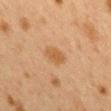{
  "biopsy_status": "not biopsied; imaged during a skin examination",
  "site": "back",
  "patient": {
    "sex": "female",
    "age_approx": 40
  },
  "image": {
    "source": "total-body photography crop",
    "field_of_view_mm": 15
  },
  "lesion_size": {
    "long_diameter_mm_approx": 3.5
  },
  "lighting": "cross-polarized"
}